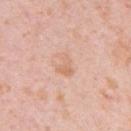Impression:
This lesion was catalogued during total-body skin photography and was not selected for biopsy.
Acquisition and patient details:
A 15 mm close-up extracted from a 3D total-body photography capture. The tile uses white-light illumination. The patient is a female in their 40s. The lesion is located on the left upper arm. The lesion's longest dimension is about 3 mm. Automated tile analysis of the lesion measured a footprint of about 4 mm² and two-axis asymmetry of about 0.4. The software also gave border irregularity of about 4.5 on a 0–10 scale, a within-lesion color-variation index near 1.5/10, and a peripheral color-asymmetry measure near 0.5.